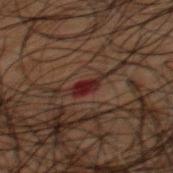The lesion was tiled from a total-body skin photograph and was not biopsied.
The subject is a male about 50 years old.
The tile uses cross-polarized illumination.
The lesion's longest dimension is about 3 mm.
From the back.
A roughly 15 mm field-of-view crop from a total-body skin photograph.